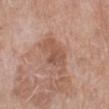Findings:
* workup — catalogued during a skin exam; not biopsied
* patient — female, aged approximately 55
* image source — 15 mm crop, total-body photography
* anatomic site — the left lower leg
* automated metrics — an eccentricity of roughly 0.85 and a shape-asymmetry score of about 0.35 (0 = symmetric); about 8 CIELAB-L* units darker than the surrounding skin and a normalized border contrast of about 6; an automated nevus-likeness rating near 0 out of 100
* lighting — white-light